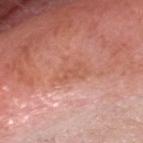Clinical impression:
Recorded during total-body skin imaging; not selected for excision or biopsy.
Clinical summary:
A male subject, roughly 60 years of age. Automated image analysis of the tile measured a footprint of about 7.5 mm², an outline eccentricity of about 0.75 (0 = round, 1 = elongated), and a symmetry-axis asymmetry near 0.45. It also reported a mean CIELAB color near L≈58 a*≈27 b*≈32, roughly 6 lightness units darker than nearby skin, and a normalized border contrast of about 5. Cropped from a total-body skin-imaging series; the visible field is about 15 mm. Measured at roughly 3.5 mm in maximum diameter. This is a white-light tile. The lesion is on the head or neck.